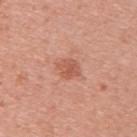follow-up: total-body-photography surveillance lesion; no biopsy
patient: female, about 30 years old
acquisition: 15 mm crop, total-body photography
location: the upper back